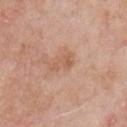This image is a 15 mm lesion crop taken from a total-body photograph. The lesion is on the right upper arm. The recorded lesion diameter is about 3 mm. Imaged with white-light lighting. A male patient, in their 60s. The total-body-photography lesion software estimated a detector confidence of about 100 out of 100 that the crop contains a lesion.